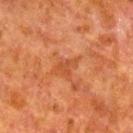follow-up: catalogued during a skin exam; not biopsied | image source: 15 mm crop, total-body photography | patient: male, aged around 80 | tile lighting: cross-polarized illumination | location: the leg | TBP lesion metrics: an average lesion color of about L≈40 a*≈25 b*≈34 (CIELAB), roughly 6 lightness units darker than nearby skin, and a lesion-to-skin contrast of about 5.5 (normalized; higher = more distinct); a border-irregularity index near 6/10, a within-lesion color-variation index near 0/10, and peripheral color asymmetry of about 0.